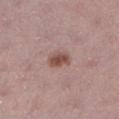– workup — total-body-photography surveillance lesion; no biopsy
– imaging modality — total-body-photography crop, ~15 mm field of view
– body site — the left lower leg
– patient — female, aged approximately 25